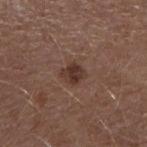{"biopsy_status": "not biopsied; imaged during a skin examination", "patient": {"sex": "male", "age_approx": 55}, "lesion_size": {"long_diameter_mm_approx": 3.0}, "image": {"source": "total-body photography crop", "field_of_view_mm": 15}, "site": "right forearm", "lighting": "white-light"}